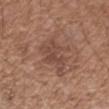The lesion was tiled from a total-body skin photograph and was not biopsied. This is a white-light tile. A 15 mm close-up extracted from a 3D total-body photography capture. The patient is a male aged approximately 50. The lesion is located on the right forearm.